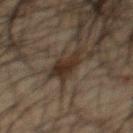Impression:
The lesion was tiled from a total-body skin photograph and was not biopsied.
Background:
On the chest. Automated tile analysis of the lesion measured a lesion area of about 7.5 mm² and a shape-asymmetry score of about 0.45 (0 = symmetric). The analysis additionally found lesion-presence confidence of about 95/100. Measured at roughly 4 mm in maximum diameter. A male subject, in their mid- to late 60s. A region of skin cropped from a whole-body photographic capture, roughly 15 mm wide. This is a cross-polarized tile.